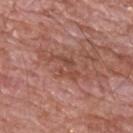biopsy_status: not biopsied; imaged during a skin examination
lighting: white-light
image:
  source: total-body photography crop
  field_of_view_mm: 15
lesion_size:
  long_diameter_mm_approx: 4.5
patient:
  sex: male
  age_approx: 60
site: upper back